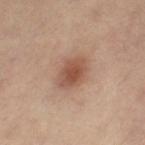This lesion was catalogued during total-body skin photography and was not selected for biopsy.
Located on the right leg.
Captured under cross-polarized illumination.
The subject is a female in their 40s.
The total-body-photography lesion software estimated a within-lesion color-variation index near 3.5/10 and radial color variation of about 1. It also reported a nevus-likeness score of about 90/100 and a lesion-detection confidence of about 100/100.
A region of skin cropped from a whole-body photographic capture, roughly 15 mm wide.
About 4 mm across.